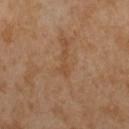Imaged during a routine full-body skin examination; the lesion was not biopsied and no histopathology is available. A lesion tile, about 15 mm wide, cut from a 3D total-body photograph. Measured at roughly 3.5 mm in maximum diameter. Automated tile analysis of the lesion measured a lesion area of about 3 mm² and a shape eccentricity near 0.95. And it measured a lesion color around L≈46 a*≈18 b*≈33 in CIELAB and roughly 5 lightness units darker than nearby skin. A male subject, in their mid-60s. Captured under cross-polarized illumination.